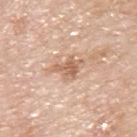The lesion is on the upper back. Captured under white-light illumination. Cropped from a total-body skin-imaging series; the visible field is about 15 mm. Longest diameter approximately 4 mm. A male patient, roughly 80 years of age. The total-body-photography lesion software estimated a lesion area of about 6.5 mm² and a shape-asymmetry score of about 0.45 (0 = symmetric). The software also gave a mean CIELAB color near L≈63 a*≈20 b*≈31 and a lesion–skin lightness drop of about 11. The software also gave border irregularity of about 5 on a 0–10 scale and a within-lesion color-variation index near 3.5/10. It also reported a classifier nevus-likeness of about 0/100 and a lesion-detection confidence of about 100/100.